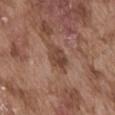Findings:
* notes: no biopsy performed (imaged during a skin exam)
* imaging modality: 15 mm crop, total-body photography
* tile lighting: white-light
* TBP lesion metrics: a nevus-likeness score of about 0/100
* body site: the abdomen
* lesion size: about 3.5 mm
* patient: male, aged approximately 75